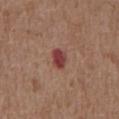Captured during whole-body skin photography for melanoma surveillance; the lesion was not biopsied. From the abdomen. A male patient about 75 years old. The lesion's longest dimension is about 2.5 mm. The tile uses white-light illumination. This image is a 15 mm lesion crop taken from a total-body photograph.